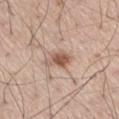| field | value |
|---|---|
| follow-up | total-body-photography surveillance lesion; no biopsy |
| patient | male, aged 58 to 62 |
| image | ~15 mm tile from a whole-body skin photo |
| site | the right thigh |
| diameter | about 3.5 mm |
| lighting | white-light |